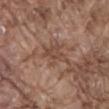- notes — catalogued during a skin exam; not biopsied
- acquisition — ~15 mm tile from a whole-body skin photo
- tile lighting — white-light illumination
- patient — male, aged 78–82
- lesion diameter — ≈4.5 mm
- location — the front of the torso